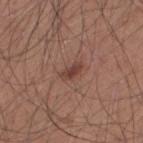Impression:
This lesion was catalogued during total-body skin photography and was not selected for biopsy.
Clinical summary:
The recorded lesion diameter is about 2.5 mm. On the upper back. A 15 mm close-up tile from a total-body photography series done for melanoma screening. Automated tile analysis of the lesion measured a border-irregularity index near 2.5/10, a color-variation rating of about 0/10, and a peripheral color-asymmetry measure near 0. The subject is a male roughly 35 years of age.